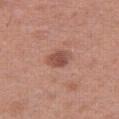The lesion was photographed on a routine skin check and not biopsied; there is no pathology result. Located on the right thigh. This image is a 15 mm lesion crop taken from a total-body photograph. A female subject, aged 38–42. Imaged with white-light lighting.